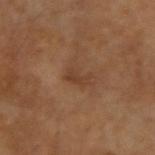follow-up: catalogued during a skin exam; not biopsied | subject: male, approximately 65 years of age | image source: total-body-photography crop, ~15 mm field of view | diameter: ≈2.5 mm | automated metrics: a lesion area of about 3 mm², an outline eccentricity of about 0.85 (0 = round, 1 = elongated), and a shape-asymmetry score of about 0.35 (0 = symmetric); a mean CIELAB color near L≈38 a*≈19 b*≈30, about 6 CIELAB-L* units darker than the surrounding skin, and a normalized border contrast of about 6; border irregularity of about 4 on a 0–10 scale, a color-variation rating of about 1/10, and radial color variation of about 0.5; lesion-presence confidence of about 100/100 | tile lighting: cross-polarized | body site: the left arm.